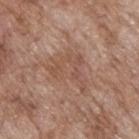lesion diameter — ~5.5 mm (longest diameter) | imaging modality — total-body-photography crop, ~15 mm field of view | anatomic site — the upper back | illumination — white-light illumination | patient — male, aged approximately 70.